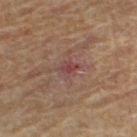Impression:
Recorded during total-body skin imaging; not selected for excision or biopsy.
Image and clinical context:
The lesion-visualizer software estimated a color-variation rating of about 2.5/10 and radial color variation of about 1. It also reported an automated nevus-likeness rating near 0 out of 100 and a detector confidence of about 95 out of 100 that the crop contains a lesion. This image is a 15 mm lesion crop taken from a total-body photograph. The lesion is located on the left lower leg. A male patient, in their mid-60s. This is a cross-polarized tile. Measured at roughly 2.5 mm in maximum diameter.